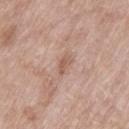Case summary:
• diameter — ~2.5 mm (longest diameter)
• image-analysis metrics — roughly 8 lightness units darker than nearby skin and a lesion-to-skin contrast of about 6 (normalized; higher = more distinct); a within-lesion color-variation index near 1/10; a nevus-likeness score of about 0/100 and lesion-presence confidence of about 100/100
• patient — female, approximately 75 years of age
• image — ~15 mm crop, total-body skin-cancer survey
• body site — the left thigh
• tile lighting — white-light illumination